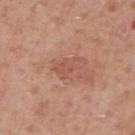workup = total-body-photography surveillance lesion; no biopsy | tile lighting = white-light illumination | image source = ~15 mm crop, total-body skin-cancer survey | subject = male, about 50 years old | anatomic site = the right upper arm | lesion size = ~3.5 mm (longest diameter).